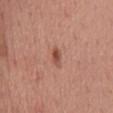The lesion was tiled from a total-body skin photograph and was not biopsied. A female subject, about 50 years old. On the mid back. A region of skin cropped from a whole-body photographic capture, roughly 15 mm wide.